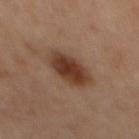follow-up = total-body-photography surveillance lesion; no biopsy | acquisition = 15 mm crop, total-body photography | tile lighting = cross-polarized illumination | anatomic site = the back.